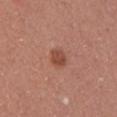lighting: white-light | lesion size: ≈2.5 mm | imaging modality: 15 mm crop, total-body photography | site: the right upper arm | automated metrics: an outline eccentricity of about 0.45 (0 = round, 1 = elongated); a border-irregularity rating of about 1.5/10 and internal color variation of about 2.5 on a 0–10 scale | subject: female, in their 20s.